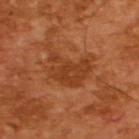The lesion was photographed on a routine skin check and not biopsied; there is no pathology result. A roughly 15 mm field-of-view crop from a total-body skin photograph. The patient is a male aged approximately 65. Imaged with cross-polarized lighting.